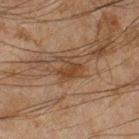Image and clinical context: Measured at roughly 3.5 mm in maximum diameter. Captured under cross-polarized illumination. From the right lower leg. The total-body-photography lesion software estimated a lesion area of about 5.5 mm² and an eccentricity of roughly 0.7. It also reported an average lesion color of about L≈34 a*≈16 b*≈27 (CIELAB), roughly 7 lightness units darker than nearby skin, and a lesion-to-skin contrast of about 7 (normalized; higher = more distinct). It also reported a classifier nevus-likeness of about 0/100. Cropped from a total-body skin-imaging series; the visible field is about 15 mm. A male subject about 45 years old.